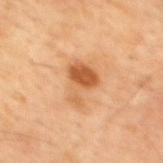Findings:
* acquisition — total-body-photography crop, ~15 mm field of view
* subject — male, in their mid-40s
* site — the upper back